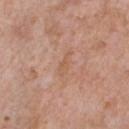This lesion was catalogued during total-body skin photography and was not selected for biopsy. Located on the chest. A 15 mm crop from a total-body photograph taken for skin-cancer surveillance. Approximately 3.5 mm at its widest. The patient is a male approximately 60 years of age.